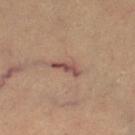notes — no biopsy performed (imaged during a skin exam) | lesion diameter — ~3.5 mm (longest diameter) | acquisition — total-body-photography crop, ~15 mm field of view | subject — aged around 60 | lighting — cross-polarized | TBP lesion metrics — a mean CIELAB color near L≈49 a*≈20 b*≈24, about 10 CIELAB-L* units darker than the surrounding skin, and a normalized lesion–skin contrast near 8 | anatomic site — the left thigh.